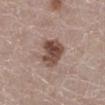Q: Was a biopsy performed?
A: catalogued during a skin exam; not biopsied
Q: Lesion location?
A: the right lower leg
Q: What are the patient's age and sex?
A: female, in their 50s
Q: How large is the lesion?
A: ≈3.5 mm
Q: Illumination type?
A: white-light illumination
Q: What is the imaging modality?
A: 15 mm crop, total-body photography